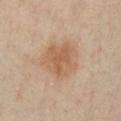biopsy_status: not biopsied; imaged during a skin examination
patient:
  sex: male
  age_approx: 65
site: chest
lighting: cross-polarized
image:
  source: total-body photography crop
  field_of_view_mm: 15